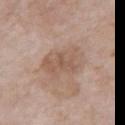diameter = about 5 mm
lighting = white-light illumination
body site = the chest
image = ~15 mm crop, total-body skin-cancer survey
subject = female, aged around 85 or older